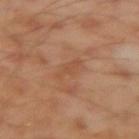workup: total-body-photography surveillance lesion; no biopsy | image-analysis metrics: a lesion color around L≈49 a*≈22 b*≈33 in CIELAB, about 5 CIELAB-L* units darker than the surrounding skin, and a lesion-to-skin contrast of about 4.5 (normalized; higher = more distinct) | body site: the right upper arm | imaging modality: ~15 mm tile from a whole-body skin photo | size: about 3.5 mm | patient: male, aged approximately 45 | illumination: cross-polarized illumination.